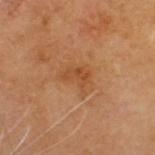Captured during whole-body skin photography for melanoma surveillance; the lesion was not biopsied.
A 15 mm close-up tile from a total-body photography series done for melanoma screening.
About 4 mm across.
Imaged with cross-polarized lighting.
A male patient about 85 years old or older.
The lesion is on the head or neck.